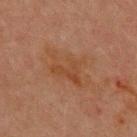Part of a total-body skin-imaging series; this lesion was reviewed on a skin check and was not flagged for biopsy. Cropped from a whole-body photographic skin survey; the tile spans about 15 mm. The lesion is located on the chest. A male subject approximately 70 years of age.